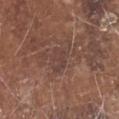Case summary:
* workup · catalogued during a skin exam; not biopsied
* imaging modality · total-body-photography crop, ~15 mm field of view
* patient · male, aged 78–82
* anatomic site · the head or neck
* diameter · ~3 mm (longest diameter)
* lighting · white-light illumination